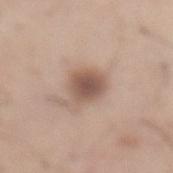The lesion is located on the abdomen. A 15 mm close-up tile from a total-body photography series done for melanoma screening. Captured under white-light illumination. A male patient in their mid- to late 60s. The total-body-photography lesion software estimated a normalized lesion–skin contrast near 8.5. And it measured a border-irregularity rating of about 2.5/10 and peripheral color asymmetry of about 1. It also reported a nevus-likeness score of about 75/100 and lesion-presence confidence of about 100/100. About 3.5 mm across.